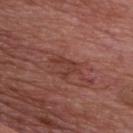On the front of the torso. The total-body-photography lesion software estimated an average lesion color of about L≈39 a*≈25 b*≈25 (CIELAB), about 7 CIELAB-L* units darker than the surrounding skin, and a lesion-to-skin contrast of about 6 (normalized; higher = more distinct). The analysis additionally found a nevus-likeness score of about 0/100 and a lesion-detection confidence of about 75/100. A 15 mm close-up extracted from a 3D total-body photography capture. A male subject aged 73–77. Longest diameter approximately 3.5 mm. Captured under white-light illumination.